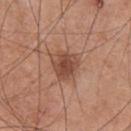Clinical impression: Captured during whole-body skin photography for melanoma surveillance; the lesion was not biopsied. Acquisition and patient details: A 15 mm crop from a total-body photograph taken for skin-cancer surveillance. Longest diameter approximately 3.5 mm. The lesion is on the chest. A male patient, aged around 55. This is a white-light tile.